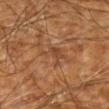<case>
<site>left forearm</site>
<patient>
  <age_approx>65</age_approx>
</patient>
<automated_metrics>
  <area_mm2_approx>5.0</area_mm2_approx>
  <eccentricity>0.6</eccentricity>
  <shape_asymmetry>0.5</shape_asymmetry>
  <cielab_L>45</cielab_L>
  <cielab_a>22</cielab_a>
  <cielab_b>34</cielab_b>
  <vs_skin_contrast_norm>5.5</vs_skin_contrast_norm>
  <color_variation_0_10>2.0</color_variation_0_10>
</automated_metrics>
<image>
  <source>total-body photography crop</source>
  <field_of_view_mm>15</field_of_view_mm>
</image>
</case>